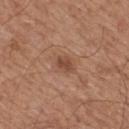Cropped from a total-body skin-imaging series; the visible field is about 15 mm.
The lesion's longest dimension is about 2.5 mm.
A male patient aged approximately 65.
Located on the mid back.